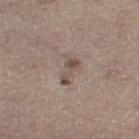Impression: Imaged during a routine full-body skin examination; the lesion was not biopsied and no histopathology is available. Background: Captured under white-light illumination. A lesion tile, about 15 mm wide, cut from a 3D total-body photograph. The lesion is located on the left thigh. A male subject, aged 68 to 72. About 3.5 mm across.